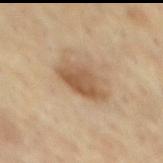Clinical impression:
Captured during whole-body skin photography for melanoma surveillance; the lesion was not biopsied.
Image and clinical context:
From the mid back. A 15 mm close-up tile from a total-body photography series done for melanoma screening. A male patient, aged 68 to 72. Approximately 5 mm at its widest. This is a cross-polarized tile. Automated image analysis of the tile measured a border-irregularity index near 2.5/10, a within-lesion color-variation index near 4/10, and a peripheral color-asymmetry measure near 1.5.